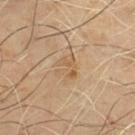Captured during whole-body skin photography for melanoma surveillance; the lesion was not biopsied. Automated tile analysis of the lesion measured border irregularity of about 5.5 on a 0–10 scale and a peripheral color-asymmetry measure near 1.5. A 15 mm crop from a total-body photograph taken for skin-cancer surveillance. Located on the chest. A male patient, aged approximately 55. This is a cross-polarized tile.